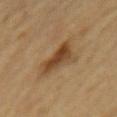Assessment: Imaged during a routine full-body skin examination; the lesion was not biopsied and no histopathology is available. Clinical summary: Captured under cross-polarized illumination. On the abdomen. A 15 mm close-up tile from a total-body photography series done for melanoma screening. Automated image analysis of the tile measured an area of roughly 8.5 mm², an eccentricity of roughly 0.65, and two-axis asymmetry of about 0.35. The software also gave a lesion color around L≈36 a*≈16 b*≈30 in CIELAB. It also reported a border-irregularity index near 3.5/10, a within-lesion color-variation index near 4.5/10, and a peripheral color-asymmetry measure near 1.5. The patient is a male approximately 85 years of age or older.